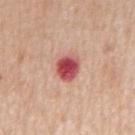Impression:
The lesion was tiled from a total-body skin photograph and was not biopsied.
Clinical summary:
Located on the front of the torso. Imaged with white-light lighting. A roughly 15 mm field-of-view crop from a total-body skin photograph. A male subject, in their 70s.